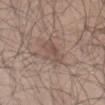Imaged during a routine full-body skin examination; the lesion was not biopsied and no histopathology is available.
From the left thigh.
A 15 mm close-up tile from a total-body photography series done for melanoma screening.
The patient is a male in their mid- to late 40s.
Automated image analysis of the tile measured a lesion area of about 7.5 mm² and an eccentricity of roughly 0.85. The analysis additionally found a border-irregularity rating of about 3/10 and peripheral color asymmetry of about 1.
The tile uses white-light illumination.
Approximately 4 mm at its widest.